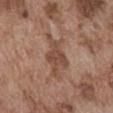workup: no biopsy performed (imaged during a skin exam)
body site: the abdomen
lesion diameter: ≈6 mm
lighting: white-light
subject: male, aged approximately 75
image source: ~15 mm tile from a whole-body skin photo
image-analysis metrics: roughly 9 lightness units darker than nearby skin and a normalized lesion–skin contrast near 6.5; an automated nevus-likeness rating near 0 out of 100 and lesion-presence confidence of about 70/100